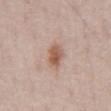lesion diameter=about 3 mm | site=the chest | lighting=white-light illumination | subject=male, approximately 50 years of age | acquisition=15 mm crop, total-body photography.